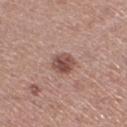Clinical summary:
A lesion tile, about 15 mm wide, cut from a 3D total-body photograph. A male patient about 50 years old. The recorded lesion diameter is about 2.5 mm. The lesion is on the left lower leg. Automated tile analysis of the lesion measured a border-irregularity rating of about 1/10, internal color variation of about 4 on a 0–10 scale, and radial color variation of about 1.5. And it measured a nevus-likeness score of about 70/100 and a lesion-detection confidence of about 100/100. Captured under white-light illumination.